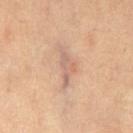notes: total-body-photography surveillance lesion; no biopsy | illumination: cross-polarized | site: the leg | automated metrics: a lesion color around L≈64 a*≈19 b*≈28 in CIELAB, a lesion–skin lightness drop of about 9, and a normalized lesion–skin contrast near 6; border irregularity of about 6.5 on a 0–10 scale, internal color variation of about 4 on a 0–10 scale, and peripheral color asymmetry of about 1.5; a classifier nevus-likeness of about 0/100 and lesion-presence confidence of about 85/100 | lesion size: ~5 mm (longest diameter) | imaging modality: ~15 mm crop, total-body skin-cancer survey | subject: female, approximately 40 years of age.